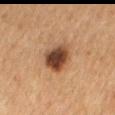{
  "biopsy_status": "not biopsied; imaged during a skin examination"
}